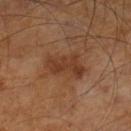follow-up — imaged on a skin check; not biopsied | subject — male, aged 63–67 | TBP lesion metrics — a lesion color around L≈36 a*≈21 b*≈31 in CIELAB, a lesion–skin lightness drop of about 8, and a normalized lesion–skin contrast near 7; a border-irregularity index near 4/10, internal color variation of about 2.5 on a 0–10 scale, and a peripheral color-asymmetry measure near 1; a nevus-likeness score of about 5/100 and a lesion-detection confidence of about 100/100 | acquisition — 15 mm crop, total-body photography | site — the left lower leg | lesion diameter — ≈4.5 mm.